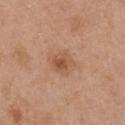* follow-up: catalogued during a skin exam; not biopsied
* tile lighting: white-light
* lesion diameter: about 3 mm
* acquisition: ~15 mm crop, total-body skin-cancer survey
* anatomic site: the chest
* subject: female, aged 38–42